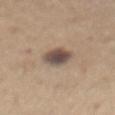| key | value |
|---|---|
| biopsy status | catalogued during a skin exam; not biopsied |
| image source | ~15 mm tile from a whole-body skin photo |
| lesion size | ≈3 mm |
| tile lighting | white-light |
| automated lesion analysis | an area of roughly 7 mm², an eccentricity of roughly 0.6, and a symmetry-axis asymmetry near 0.15; a border-irregularity rating of about 1.5/10 and peripheral color asymmetry of about 1.5; a classifier nevus-likeness of about 25/100 and a detector confidence of about 100 out of 100 that the crop contains a lesion |
| patient | male, aged 63 to 67 |
| body site | the chest |